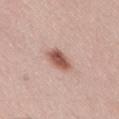follow-up = total-body-photography surveillance lesion; no biopsy | lighting = white-light illumination | lesion diameter = ≈3.5 mm | location = the right thigh | patient = male, in their mid-50s | acquisition = ~15 mm tile from a whole-body skin photo.